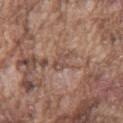  biopsy_status: not biopsied; imaged during a skin examination
  lighting: white-light
  automated_metrics:
    area_mm2_approx: 4.0
    eccentricity: 0.8
    shape_asymmetry: 0.4
    cielab_L: 49
    cielab_a: 18
    cielab_b: 25
    vs_skin_darker_L: 7.0
    vs_skin_contrast_norm: 5.5
    border_irregularity_0_10: 5.0
    color_variation_0_10: 1.5
    peripheral_color_asymmetry: 0.5
  site: chest
  patient:
    sex: male
    age_approx: 75
  lesion_size:
    long_diameter_mm_approx: 3.0
  image:
    source: total-body photography crop
    field_of_view_mm: 15